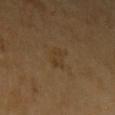| feature | finding |
|---|---|
| acquisition | 15 mm crop, total-body photography |
| location | the left upper arm |
| subject | male, approximately 65 years of age |
| image-analysis metrics | a lesion color around L≈36 a*≈14 b*≈31 in CIELAB, about 5 CIELAB-L* units darker than the surrounding skin, and a lesion-to-skin contrast of about 5 (normalized; higher = more distinct); a within-lesion color-variation index near 1.5/10 and a peripheral color-asymmetry measure near 0.5; an automated nevus-likeness rating near 5 out of 100 |